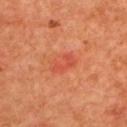Case summary:
– follow-up — imaged on a skin check; not biopsied
– body site — the upper back
– subject — female, aged 38–42
– image source — 15 mm crop, total-body photography